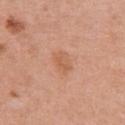{"biopsy_status": "not biopsied; imaged during a skin examination", "patient": {"sex": "female", "age_approx": 50}, "automated_metrics": {"area_mm2_approx": 4.5, "eccentricity": 0.65, "shape_asymmetry": 0.15, "cielab_L": 59, "cielab_a": 24, "cielab_b": 34, "vs_skin_darker_L": 7.0, "vs_skin_contrast_norm": 5.5, "color_variation_0_10": 2.5, "peripheral_color_asymmetry": 1.0, "nevus_likeness_0_100": 15, "lesion_detection_confidence_0_100": 100}, "image": {"source": "total-body photography crop", "field_of_view_mm": 15}, "lesion_size": {"long_diameter_mm_approx": 3.0}, "site": "chest"}